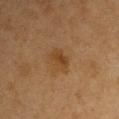| feature | finding |
|---|---|
| workup | catalogued during a skin exam; not biopsied |
| lesion size | ~3 mm (longest diameter) |
| automated lesion analysis | a footprint of about 5 mm², a shape eccentricity near 0.75, and a symmetry-axis asymmetry near 0.25; a lesion color around L≈36 a*≈17 b*≈32 in CIELAB and a lesion-to-skin contrast of about 6.5 (normalized; higher = more distinct); border irregularity of about 3 on a 0–10 scale, a within-lesion color-variation index near 4/10, and peripheral color asymmetry of about 1.5 |
| anatomic site | the left upper arm |
| subject | female, in their 40s |
| illumination | cross-polarized illumination |
| imaging modality | ~15 mm tile from a whole-body skin photo |